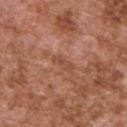Recorded during total-body skin imaging; not selected for excision or biopsy. The tile uses white-light illumination. A 15 mm close-up extracted from a 3D total-body photography capture. The lesion is on the upper back. An algorithmic analysis of the crop reported an area of roughly 2.5 mm², a shape eccentricity near 0.9, and a shape-asymmetry score of about 0.45 (0 = symmetric). It also reported a border-irregularity rating of about 5.5/10, a color-variation rating of about 0/10, and peripheral color asymmetry of about 0. The software also gave an automated nevus-likeness rating near 0 out of 100. The patient is a male aged around 75. Approximately 3 mm at its widest.